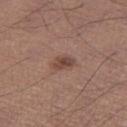The lesion was photographed on a routine skin check and not biopsied; there is no pathology result.
A 15 mm close-up extracted from a 3D total-body photography capture.
Captured under white-light illumination.
The patient is a male roughly 65 years of age.
About 3 mm across.
The lesion is located on the left thigh.